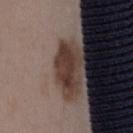Background: This is a white-light tile. From the mid back. The lesion-visualizer software estimated a footprint of about 8.5 mm², an outline eccentricity of about 0.9 (0 = round, 1 = elongated), and a shape-asymmetry score of about 0.25 (0 = symmetric). The analysis additionally found a lesion color around L≈34 a*≈16 b*≈21 in CIELAB and about 5 CIELAB-L* units darker than the surrounding skin. The analysis additionally found border irregularity of about 3.5 on a 0–10 scale, internal color variation of about 2.5 on a 0–10 scale, and a peripheral color-asymmetry measure near 1. A female subject in their mid- to late 30s. Longest diameter approximately 5 mm. A region of skin cropped from a whole-body photographic capture, roughly 15 mm wide.